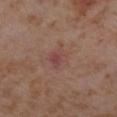workup = imaged on a skin check; not biopsied | imaging modality = ~15 mm tile from a whole-body skin photo | subject = female, about 55 years old | anatomic site = the left lower leg | tile lighting = cross-polarized.